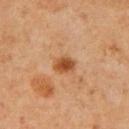Findings:
• follow-up — no biopsy performed (imaged during a skin exam)
• image — ~15 mm crop, total-body skin-cancer survey
• anatomic site — the right upper arm
• subject — male, about 60 years old